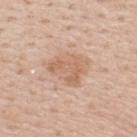• biopsy status — catalogued during a skin exam; not biopsied
• lesion diameter — ≈5 mm
• patient — male, about 60 years old
• image — ~15 mm tile from a whole-body skin photo
• illumination — white-light illumination
• location — the upper back